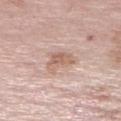Clinical impression: Recorded during total-body skin imaging; not selected for excision or biopsy. Clinical summary: A region of skin cropped from a whole-body photographic capture, roughly 15 mm wide. From the right lower leg. Imaged with white-light lighting. A female subject roughly 70 years of age. Approximately 3.5 mm at its widest.